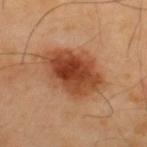This lesion was catalogued during total-body skin photography and was not selected for biopsy. An algorithmic analysis of the crop reported a lesion color around L≈46 a*≈26 b*≈35 in CIELAB and about 14 CIELAB-L* units darker than the surrounding skin. It also reported an automated nevus-likeness rating near 100 out of 100 and a detector confidence of about 100 out of 100 that the crop contains a lesion. A lesion tile, about 15 mm wide, cut from a 3D total-body photograph. This is a cross-polarized tile. A male subject, aged around 40. The lesion is located on the upper back.